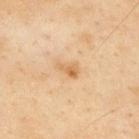Assessment:
The lesion was photographed on a routine skin check and not biopsied; there is no pathology result.
Clinical summary:
The subject is a male aged 53 to 57. The lesion-visualizer software estimated a lesion area of about 3 mm², an outline eccentricity of about 0.8 (0 = round, 1 = elongated), and a shape-asymmetry score of about 0.45 (0 = symmetric). The software also gave internal color variation of about 3.5 on a 0–10 scale and a peripheral color-asymmetry measure near 1.5. The tile uses cross-polarized illumination. The lesion is on the upper back. Cropped from a total-body skin-imaging series; the visible field is about 15 mm. The recorded lesion diameter is about 2.5 mm.